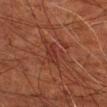{
  "biopsy_status": "not biopsied; imaged during a skin examination",
  "patient": {
    "sex": "male",
    "age_approx": 80
  },
  "image": {
    "source": "total-body photography crop",
    "field_of_view_mm": 15
  },
  "automated_metrics": {
    "area_mm2_approx": 6.5,
    "eccentricity": 0.8,
    "cielab_L": 27,
    "cielab_a": 22,
    "cielab_b": 23,
    "vs_skin_darker_L": 5.0,
    "border_irregularity_0_10": 3.5,
    "peripheral_color_asymmetry": 0.5,
    "nevus_likeness_0_100": 0,
    "lesion_detection_confidence_0_100": 95
  },
  "lighting": "cross-polarized",
  "site": "left upper arm"
}